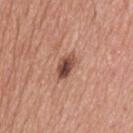No biopsy was performed on this lesion — it was imaged during a full skin examination and was not determined to be concerning. A male patient, in their mid- to late 60s. Measured at roughly 3 mm in maximum diameter. The lesion is located on the upper back. An algorithmic analysis of the crop reported an average lesion color of about L≈47 a*≈23 b*≈27 (CIELAB). It also reported an automated nevus-likeness rating near 55 out of 100 and a detector confidence of about 100 out of 100 that the crop contains a lesion. A roughly 15 mm field-of-view crop from a total-body skin photograph.